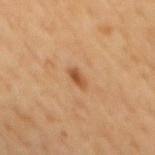Findings:
- biopsy status · total-body-photography surveillance lesion; no biopsy
- patient · male, aged 58–62
- body site · the mid back
- image source · ~15 mm tile from a whole-body skin photo